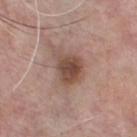No biopsy was performed on this lesion — it was imaged during a full skin examination and was not determined to be concerning.
Located on the chest.
Automated tile analysis of the lesion measured a lesion color around L≈48 a*≈19 b*≈25 in CIELAB, about 12 CIELAB-L* units darker than the surrounding skin, and a normalized lesion–skin contrast near 9. The software also gave a classifier nevus-likeness of about 75/100.
Longest diameter approximately 4 mm.
A male patient about 55 years old.
A close-up tile cropped from a whole-body skin photograph, about 15 mm across.
This is a white-light tile.